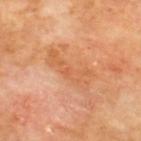Imaged during a routine full-body skin examination; the lesion was not biopsied and no histopathology is available. Imaged with cross-polarized lighting. Measured at roughly 6 mm in maximum diameter. A roughly 15 mm field-of-view crop from a total-body skin photograph. A male patient aged 68–72. Located on the upper back. The lesion-visualizer software estimated an area of roughly 10 mm². The software also gave border irregularity of about 5.5 on a 0–10 scale, a within-lesion color-variation index near 3/10, and peripheral color asymmetry of about 1.